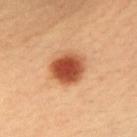Clinical impression:
This lesion was catalogued during total-body skin photography and was not selected for biopsy.
Acquisition and patient details:
Imaged with cross-polarized lighting. This image is a 15 mm lesion crop taken from a total-body photograph. From the arm. About 4.5 mm across. A male patient aged approximately 40.